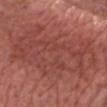<case>
  <biopsy_status>not biopsied; imaged during a skin examination</biopsy_status>
  <patient>
    <sex>male</sex>
    <age_approx>65</age_approx>
  </patient>
  <image>
    <source>total-body photography crop</source>
    <field_of_view_mm>15</field_of_view_mm>
  </image>
  <lesion_size>
    <long_diameter_mm_approx>12.0</long_diameter_mm_approx>
  </lesion_size>
  <site>front of the torso</site>
</case>